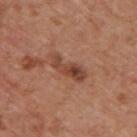Q: Is there a histopathology result?
A: no biopsy performed (imaged during a skin exam)
Q: Patient demographics?
A: male, in their mid-60s
Q: What lighting was used for the tile?
A: white-light
Q: What is the imaging modality?
A: ~15 mm tile from a whole-body skin photo
Q: Lesion location?
A: the upper back
Q: Automated lesion metrics?
A: roughly 10 lightness units darker than nearby skin and a normalized lesion–skin contrast near 8